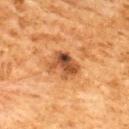A male subject aged approximately 60. A region of skin cropped from a whole-body photographic capture, roughly 15 mm wide. The lesion is on the back.